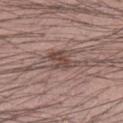The lesion was tiled from a total-body skin photograph and was not biopsied.